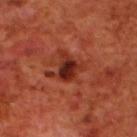Imaged during a routine full-body skin examination; the lesion was not biopsied and no histopathology is available.
This is a cross-polarized tile.
The total-body-photography lesion software estimated a footprint of about 6 mm², an eccentricity of roughly 0.6, and two-axis asymmetry of about 0.35. And it measured a lesion color around L≈29 a*≈30 b*≈30 in CIELAB, a lesion–skin lightness drop of about 12, and a lesion-to-skin contrast of about 11 (normalized; higher = more distinct). It also reported a border-irregularity index near 3.5/10, a color-variation rating of about 6/10, and a peripheral color-asymmetry measure near 2.
A 15 mm close-up tile from a total-body photography series done for melanoma screening.
A male patient, aged 68 to 72.
The lesion is on the upper back.